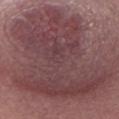<record>
<biopsy_status>not biopsied; imaged during a skin examination</biopsy_status>
<image>
  <source>total-body photography crop</source>
  <field_of_view_mm>15</field_of_view_mm>
</image>
<automated_metrics>
  <area_mm2_approx>130.0</area_mm2_approx>
  <shape_asymmetry>0.35</shape_asymmetry>
</automated_metrics>
<lesion_size>
  <long_diameter_mm_approx>17.0</long_diameter_mm_approx>
</lesion_size>
<patient>
  <sex>female</sex>
  <age_approx>55</age_approx>
</patient>
<site>chest</site>
</record>